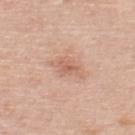Imaged during a routine full-body skin examination; the lesion was not biopsied and no histopathology is available.
A roughly 15 mm field-of-view crop from a total-body skin photograph.
A male subject, about 50 years old.
Approximately 4 mm at its widest.
Imaged with white-light lighting.
Located on the upper back.